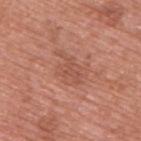Assessment:
The lesion was photographed on a routine skin check and not biopsied; there is no pathology result.
Acquisition and patient details:
Approximately 4.5 mm at its widest. On the upper back. A 15 mm close-up extracted from a 3D total-body photography capture. The tile uses white-light illumination. A male patient, aged 68 to 72.